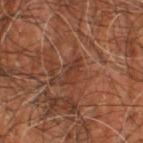Impression: The lesion was tiled from a total-body skin photograph and was not biopsied. Acquisition and patient details: About 3.5 mm across. A male patient roughly 60 years of age. A 15 mm close-up tile from a total-body photography series done for melanoma screening. An algorithmic analysis of the crop reported a mean CIELAB color near L≈35 a*≈22 b*≈27. The software also gave a classifier nevus-likeness of about 0/100 and a lesion-detection confidence of about 55/100. The lesion is on the right leg.